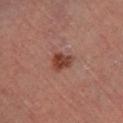– follow-up · catalogued during a skin exam; not biopsied
– subject · male, roughly 65 years of age
– body site · the right lower leg
– image · 15 mm crop, total-body photography